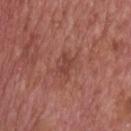No biopsy was performed on this lesion — it was imaged during a full skin examination and was not determined to be concerning. Located on the upper back. A lesion tile, about 15 mm wide, cut from a 3D total-body photograph. Imaged with white-light lighting. A male subject, aged approximately 75. The recorded lesion diameter is about 3 mm.